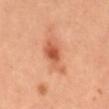Assessment: Recorded during total-body skin imaging; not selected for excision or biopsy. Context: The lesion is on the mid back. The lesion's longest dimension is about 5.5 mm. The subject is female. Imaged with cross-polarized lighting. A roughly 15 mm field-of-view crop from a total-body skin photograph.